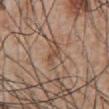{"biopsy_status": "not biopsied; imaged during a skin examination", "patient": {"sex": "male", "age_approx": 55}, "image": {"source": "total-body photography crop", "field_of_view_mm": 15}, "lighting": "white-light", "lesion_size": {"long_diameter_mm_approx": 3.0}, "site": "abdomen"}